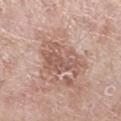The lesion was tiled from a total-body skin photograph and was not biopsied. This is a white-light tile. Cropped from a whole-body photographic skin survey; the tile spans about 15 mm. A female subject, aged 68 to 72. The lesion is on the left lower leg.